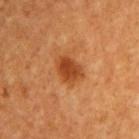Q: Who is the patient?
A: male, in their 60s
Q: Where on the body is the lesion?
A: the front of the torso
Q: How was the tile lit?
A: cross-polarized
Q: Lesion size?
A: ~3.5 mm (longest diameter)
Q: What kind of image is this?
A: 15 mm crop, total-body photography